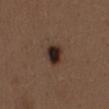The lesion was photographed on a routine skin check and not biopsied; there is no pathology result. Located on the chest. Measured at roughly 3.5 mm in maximum diameter. Automated image analysis of the tile measured a mean CIELAB color near L≈27 a*≈15 b*≈20, about 14 CIELAB-L* units darker than the surrounding skin, and a lesion-to-skin contrast of about 14 (normalized; higher = more distinct). The analysis additionally found border irregularity of about 1.5 on a 0–10 scale and peripheral color asymmetry of about 2. A lesion tile, about 15 mm wide, cut from a 3D total-body photograph. A female subject, aged 38–42. Captured under white-light illumination.